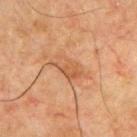Impression: Recorded during total-body skin imaging; not selected for excision or biopsy. Acquisition and patient details: This is a cross-polarized tile. A close-up tile cropped from a whole-body skin photograph, about 15 mm across. The lesion is located on the front of the torso. A male subject, roughly 65 years of age.